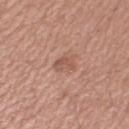Impression:
No biopsy was performed on this lesion — it was imaged during a full skin examination and was not determined to be concerning.
Acquisition and patient details:
The lesion's longest dimension is about 3 mm. A male patient aged approximately 45. The tile uses white-light illumination. A 15 mm crop from a total-body photograph taken for skin-cancer surveillance. On the right upper arm.